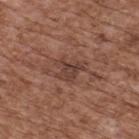biopsy status = total-body-photography surveillance lesion; no biopsy | size = about 3.5 mm | anatomic site = the upper back | patient = male, aged approximately 75 | lighting = white-light illumination | imaging modality = ~15 mm tile from a whole-body skin photo.